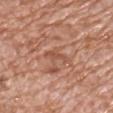image: ~15 mm tile from a whole-body skin photo | patient: male, aged around 70 | body site: the chest.